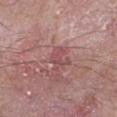This lesion was catalogued during total-body skin photography and was not selected for biopsy. The recorded lesion diameter is about 3 mm. A 15 mm close-up tile from a total-body photography series done for melanoma screening. Located on the left forearm. Captured under white-light illumination. The subject is a male aged 58 to 62.